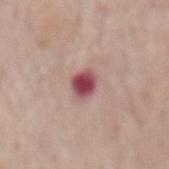| field | value |
|---|---|
| workup | imaged on a skin check; not biopsied |